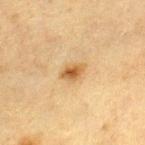Q: Was this lesion biopsied?
A: total-body-photography surveillance lesion; no biopsy
Q: Lesion size?
A: ≈3 mm
Q: Lesion location?
A: the leg
Q: How was this image acquired?
A: 15 mm crop, total-body photography
Q: Who is the patient?
A: female, aged around 55
Q: How was the tile lit?
A: cross-polarized
Q: What did automated image analysis measure?
A: a footprint of about 4.5 mm² and a shape-asymmetry score of about 0.25 (0 = symmetric); about 10 CIELAB-L* units darker than the surrounding skin; a border-irregularity rating of about 2.5/10 and a peripheral color-asymmetry measure near 1